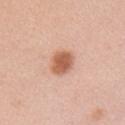Captured during whole-body skin photography for melanoma surveillance; the lesion was not biopsied.
The subject is a female in their mid-40s.
This image is a 15 mm lesion crop taken from a total-body photograph.
On the right upper arm.
Longest diameter approximately 3.5 mm.